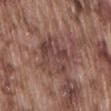notes=total-body-photography surveillance lesion; no biopsy
image source=total-body-photography crop, ~15 mm field of view
site=the lower back
patient=male, in their mid-70s
size=~6 mm (longest diameter)